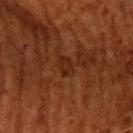No biopsy was performed on this lesion — it was imaged during a full skin examination and was not determined to be concerning. Automated image analysis of the tile measured a lesion area of about 3.5 mm² and an outline eccentricity of about 0.8 (0 = round, 1 = elongated). And it measured a mean CIELAB color near L≈21 a*≈21 b*≈26, roughly 5 lightness units darker than nearby skin, and a normalized border contrast of about 5.5. Captured under cross-polarized illumination. A male patient, in their 60s. Cropped from a total-body skin-imaging series; the visible field is about 15 mm. The lesion is on the left upper arm.